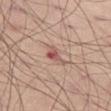<case>
<biopsy_status>not biopsied; imaged during a skin examination</biopsy_status>
<lighting>white-light</lighting>
<site>left thigh</site>
<image>
  <source>total-body photography crop</source>
  <field_of_view_mm>15</field_of_view_mm>
</image>
<automated_metrics>
  <eccentricity>0.8</eccentricity>
  <shape_asymmetry>0.35</shape_asymmetry>
  <border_irregularity_0_10>3.5</border_irregularity_0_10>
  <color_variation_0_10>9.0</color_variation_0_10>
  <peripheral_color_asymmetry>3.0</peripheral_color_asymmetry>
</automated_metrics>
<patient>
  <sex>male</sex>
  <age_approx>45</age_approx>
</patient>
</case>